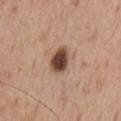Findings:
* imaging modality: total-body-photography crop, ~15 mm field of view
* tile lighting: white-light
* location: the chest
* patient: male, aged 53 to 57